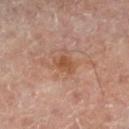Imaged during a routine full-body skin examination; the lesion was not biopsied and no histopathology is available. Located on the right lower leg. The patient is a male aged 63 to 67. Captured under cross-polarized illumination. Cropped from a whole-body photographic skin survey; the tile spans about 15 mm. The lesion-visualizer software estimated a lesion area of about 4.5 mm² and an outline eccentricity of about 0.65 (0 = round, 1 = elongated). The analysis additionally found a mean CIELAB color near L≈48 a*≈21 b*≈31, roughly 8 lightness units darker than nearby skin, and a normalized border contrast of about 7. It also reported internal color variation of about 2 on a 0–10 scale and radial color variation of about 0.5. And it measured a nevus-likeness score of about 0/100.